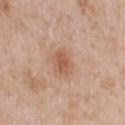<lesion>
<biopsy_status>not biopsied; imaged during a skin examination</biopsy_status>
<site>back</site>
<image>
  <source>total-body photography crop</source>
  <field_of_view_mm>15</field_of_view_mm>
</image>
<patient>
  <sex>male</sex>
  <age_approx>40</age_approx>
</patient>
<lesion_size>
  <long_diameter_mm_approx>3.5</long_diameter_mm_approx>
</lesion_size>
</lesion>